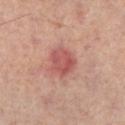Notes:
* follow-up: no biopsy performed (imaged during a skin exam)
* patient: male, in their 50s
* image source: 15 mm crop, total-body photography
* location: the left leg